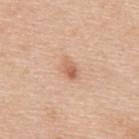Background: Located on the upper back. A male subject, aged 48–52. Cropped from a whole-body photographic skin survey; the tile spans about 15 mm.